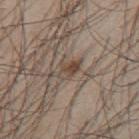biopsy_status: not biopsied; imaged during a skin examination
site: mid back
image:
  source: total-body photography crop
  field_of_view_mm: 15
lesion_size:
  long_diameter_mm_approx: 3.0
lighting: white-light
automated_metrics:
  area_mm2_approx: 4.0
  eccentricity: 0.8
  shape_asymmetry: 0.35
  nevus_likeness_0_100: 70
  lesion_detection_confidence_0_100: 85
patient:
  sex: male
  age_approx: 45